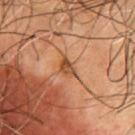Part of a total-body skin-imaging series; this lesion was reviewed on a skin check and was not flagged for biopsy. The tile uses cross-polarized illumination. The lesion is on the chest. Approximately 3 mm at its widest. The patient is a male aged 53 to 57. A lesion tile, about 15 mm wide, cut from a 3D total-body photograph.